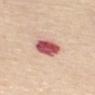Impression: Part of a total-body skin-imaging series; this lesion was reviewed on a skin check and was not flagged for biopsy. Context: This is a white-light tile. Measured at roughly 3.5 mm in maximum diameter. A female subject about 65 years old. The lesion is on the mid back. This image is a 15 mm lesion crop taken from a total-body photograph.